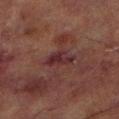Case summary:
– biopsy status — imaged on a skin check; not biopsied
– patient — male, in their 70s
– body site — the left lower leg
– image — ~15 mm tile from a whole-body skin photo
– lesion size — ~3 mm (longest diameter)